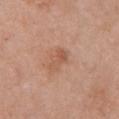Assessment:
Part of a total-body skin-imaging series; this lesion was reviewed on a skin check and was not flagged for biopsy.
Clinical summary:
A female patient, aged 58–62. The lesion is on the front of the torso. A close-up tile cropped from a whole-body skin photograph, about 15 mm across. This is a white-light tile.